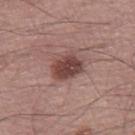notes = no biopsy performed (imaged during a skin exam) | illumination = white-light | TBP lesion metrics = an area of roughly 8.5 mm², a shape eccentricity near 0.65, and two-axis asymmetry of about 0.2; an average lesion color of about L≈43 a*≈21 b*≈22 (CIELAB) and a normalized border contrast of about 10; border irregularity of about 2 on a 0–10 scale, a within-lesion color-variation index near 4/10, and radial color variation of about 1 | subject = male, aged approximately 70 | lesion diameter = about 4 mm | imaging modality = 15 mm crop, total-body photography | anatomic site = the leg.